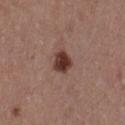follow-up = total-body-photography surveillance lesion; no biopsy | patient = male, approximately 40 years of age | location = the chest | image source = 15 mm crop, total-body photography.